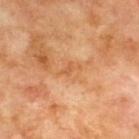Impression: The lesion was photographed on a routine skin check and not biopsied; there is no pathology result. Clinical summary: Automated tile analysis of the lesion measured a lesion area of about 2.5 mm². The software also gave a border-irregularity index near 5.5/10, a color-variation rating of about 0/10, and radial color variation of about 0. The subject is a male approximately 70 years of age. A lesion tile, about 15 mm wide, cut from a 3D total-body photograph. The lesion is on the upper back. Measured at roughly 3 mm in maximum diameter.